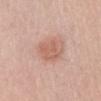notes — total-body-photography surveillance lesion; no biopsy
tile lighting — white-light illumination
subject — male, roughly 60 years of age
location — the abdomen
imaging modality — total-body-photography crop, ~15 mm field of view
automated metrics — a footprint of about 8 mm², a shape eccentricity near 0.6, and a symmetry-axis asymmetry near 0.25; an average lesion color of about L≈61 a*≈23 b*≈28 (CIELAB), a lesion–skin lightness drop of about 8, and a lesion-to-skin contrast of about 6 (normalized; higher = more distinct); a border-irregularity index near 2.5/10, internal color variation of about 2 on a 0–10 scale, and a peripheral color-asymmetry measure near 0.5
diameter — ~3.5 mm (longest diameter)